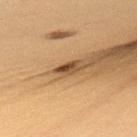Q: Was this lesion biopsied?
A: total-body-photography surveillance lesion; no biopsy
Q: Where on the body is the lesion?
A: the upper back
Q: Lesion size?
A: ≈3 mm
Q: Who is the patient?
A: male, aged around 35
Q: What is the imaging modality?
A: ~15 mm crop, total-body skin-cancer survey
Q: Automated lesion metrics?
A: a footprint of about 3 mm², an outline eccentricity of about 0.85 (0 = round, 1 = elongated), and two-axis asymmetry of about 0.35; a border-irregularity index near 3.5/10 and a color-variation rating of about 2.5/10; a nevus-likeness score of about 90/100 and a lesion-detection confidence of about 70/100